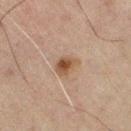Captured during whole-body skin photography for melanoma surveillance; the lesion was not biopsied.
The lesion-visualizer software estimated a lesion area of about 5 mm², an eccentricity of roughly 0.5, and a symmetry-axis asymmetry near 0.25. And it measured radial color variation of about 1. It also reported a nevus-likeness score of about 95/100 and a detector confidence of about 100 out of 100 that the crop contains a lesion.
A male subject aged 58–62.
From the left leg.
A 15 mm close-up tile from a total-body photography series done for melanoma screening.
Measured at roughly 2.5 mm in maximum diameter.
Imaged with cross-polarized lighting.